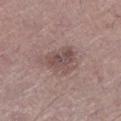biopsy_status: not biopsied; imaged during a skin examination